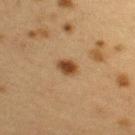Impression:
Captured during whole-body skin photography for melanoma surveillance; the lesion was not biopsied.
Clinical summary:
A 15 mm crop from a total-body photograph taken for skin-cancer surveillance. The recorded lesion diameter is about 2.5 mm. A female patient, aged approximately 40. On the right upper arm. An algorithmic analysis of the crop reported an automated nevus-likeness rating near 100 out of 100 and a detector confidence of about 100 out of 100 that the crop contains a lesion. Imaged with cross-polarized lighting.